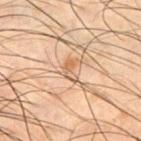<case>
  <biopsy_status>not biopsied; imaged during a skin examination</biopsy_status>
  <automated_metrics>
    <area_mm2_approx>3.0</area_mm2_approx>
    <eccentricity>0.9</eccentricity>
    <shape_asymmetry>0.45</shape_asymmetry>
    <cielab_L>48</cielab_L>
    <cielab_a>16</cielab_a>
    <cielab_b>29</cielab_b>
    <vs_skin_darker_L>7.0</vs_skin_darker_L>
    <vs_skin_contrast_norm>6.0</vs_skin_contrast_norm>
    <border_irregularity_0_10>4.5</border_irregularity_0_10>
    <peripheral_color_asymmetry>0.0</peripheral_color_asymmetry>
  </automated_metrics>
  <lesion_size>
    <long_diameter_mm_approx>3.0</long_diameter_mm_approx>
  </lesion_size>
  <site>chest</site>
  <lighting>cross-polarized</lighting>
  <patient>
    <sex>male</sex>
    <age_approx>50</age_approx>
  </patient>
  <image>
    <source>total-body photography crop</source>
    <field_of_view_mm>15</field_of_view_mm>
  </image>
</case>